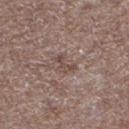No biopsy was performed on this lesion — it was imaged during a full skin examination and was not determined to be concerning.
A lesion tile, about 15 mm wide, cut from a 3D total-body photograph.
On the leg.
A male patient, aged approximately 70.